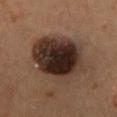<record>
<biopsy_status>not biopsied; imaged during a skin examination</biopsy_status>
</record>